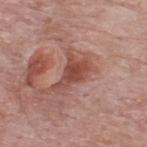The lesion was tiled from a total-body skin photograph and was not biopsied. A male patient aged approximately 55. The lesion-visualizer software estimated a border-irregularity index near 5.5/10, internal color variation of about 4 on a 0–10 scale, and peripheral color asymmetry of about 1. It also reported a classifier nevus-likeness of about 0/100. A lesion tile, about 15 mm wide, cut from a 3D total-body photograph. On the back. The tile uses white-light illumination.